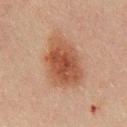The lesion was tiled from a total-body skin photograph and was not biopsied. A lesion tile, about 15 mm wide, cut from a 3D total-body photograph. The lesion is located on the chest. A male patient roughly 50 years of age.